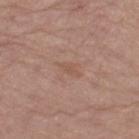Findings:
* notes — catalogued during a skin exam; not biopsied
* anatomic site — the left thigh
* image-analysis metrics — a footprint of about 2.5 mm², a shape eccentricity near 0.9, and a shape-asymmetry score of about 0.45 (0 = symmetric); a lesion color around L≈53 a*≈20 b*≈28 in CIELAB and about 6 CIELAB-L* units darker than the surrounding skin; a color-variation rating of about 0/10; a classifier nevus-likeness of about 0/100 and a detector confidence of about 100 out of 100 that the crop contains a lesion
* image source — total-body-photography crop, ~15 mm field of view
* lesion diameter — ≈3 mm
* subject — female, roughly 65 years of age
* illumination — white-light